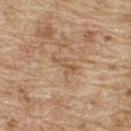The lesion was tiled from a total-body skin photograph and was not biopsied.